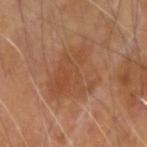Clinical impression:
No biopsy was performed on this lesion — it was imaged during a full skin examination and was not determined to be concerning.
Background:
The lesion is on the right forearm. This is a cross-polarized tile. A male subject, in their 70s. About 7 mm across. Cropped from a total-body skin-imaging series; the visible field is about 15 mm.